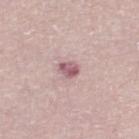No biopsy was performed on this lesion — it was imaged during a full skin examination and was not determined to be concerning. An algorithmic analysis of the crop reported a border-irregularity rating of about 2/10, a within-lesion color-variation index near 4/10, and a peripheral color-asymmetry measure near 1.5. And it measured lesion-presence confidence of about 100/100. This image is a 15 mm lesion crop taken from a total-body photograph. This is a white-light tile. A female patient, in their mid-50s. From the right lower leg.